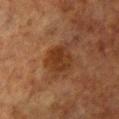Clinical impression: No biopsy was performed on this lesion — it was imaged during a full skin examination and was not determined to be concerning. Background: Cropped from a whole-body photographic skin survey; the tile spans about 15 mm. The tile uses cross-polarized illumination. The lesion's longest dimension is about 3 mm. A female patient aged approximately 55. Located on the front of the torso.